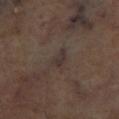Case summary:
• notes — imaged on a skin check; not biopsied
• location — the leg
• TBP lesion metrics — a footprint of about 3 mm², a shape eccentricity near 0.85, and two-axis asymmetry of about 0.45; a lesion color around L≈31 a*≈11 b*≈16 in CIELAB, about 6 CIELAB-L* units darker than the surrounding skin, and a normalized border contrast of about 7.5; a peripheral color-asymmetry measure near 0
• size — about 2.5 mm
• image source — ~15 mm crop, total-body skin-cancer survey
• lighting — cross-polarized